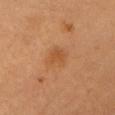Q: Was a biopsy performed?
A: imaged on a skin check; not biopsied
Q: How was this image acquired?
A: total-body-photography crop, ~15 mm field of view
Q: What is the anatomic site?
A: the head or neck
Q: Patient demographics?
A: female, aged 43 to 47